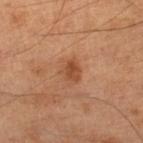Part of a total-body skin-imaging series; this lesion was reviewed on a skin check and was not flagged for biopsy.
This is a cross-polarized tile.
The subject is a male aged 63 to 67.
The total-body-photography lesion software estimated an area of roughly 4.5 mm². The software also gave an average lesion color of about L≈46 a*≈24 b*≈34 (CIELAB), about 9 CIELAB-L* units darker than the surrounding skin, and a normalized lesion–skin contrast near 7. And it measured a border-irregularity rating of about 2/10 and a color-variation rating of about 3/10. The software also gave a classifier nevus-likeness of about 50/100 and a lesion-detection confidence of about 100/100.
A region of skin cropped from a whole-body photographic capture, roughly 15 mm wide.
About 2.5 mm across.
On the right lower leg.